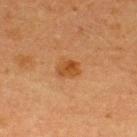Imaged during a routine full-body skin examination; the lesion was not biopsied and no histopathology is available. A 15 mm close-up tile from a total-body photography series done for melanoma screening. A male subject, about 40 years old. The lesion is on the back. The recorded lesion diameter is about 2.5 mm. The tile uses cross-polarized illumination.